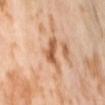The lesion was photographed on a routine skin check and not biopsied; there is no pathology result.
A female subject, about 55 years old.
The lesion's longest dimension is about 3.5 mm.
This is a cross-polarized tile.
Cropped from a total-body skin-imaging series; the visible field is about 15 mm.
The lesion is on the lower back.
Automated tile analysis of the lesion measured a mean CIELAB color near L≈60 a*≈23 b*≈37 and a normalized border contrast of about 8. And it measured a nevus-likeness score of about 0/100 and lesion-presence confidence of about 100/100.